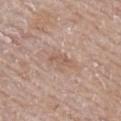| field | value |
|---|---|
| workup | imaged on a skin check; not biopsied |
| anatomic site | the right upper arm |
| image | ~15 mm crop, total-body skin-cancer survey |
| patient | male, aged around 65 |